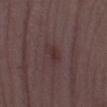Context:
Located on the left lower leg. This is a white-light tile. A female subject aged around 35. The lesion-visualizer software estimated a mean CIELAB color near L≈32 a*≈17 b*≈16, roughly 5 lightness units darker than nearby skin, and a normalized border contrast of about 6. A 15 mm close-up extracted from a 3D total-body photography capture. The recorded lesion diameter is about 3 mm.